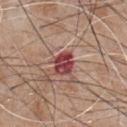Impression:
The lesion was tiled from a total-body skin photograph and was not biopsied.
Acquisition and patient details:
A lesion tile, about 15 mm wide, cut from a 3D total-body photograph. Captured under white-light illumination. Measured at roughly 3 mm in maximum diameter. A male subject, in their mid- to late 60s. An algorithmic analysis of the crop reported a mean CIELAB color near L≈43 a*≈31 b*≈22, a lesion–skin lightness drop of about 15, and a normalized lesion–skin contrast near 11.5. Located on the chest.